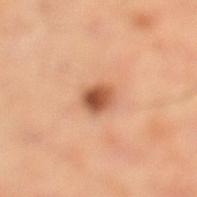biopsy status: total-body-photography surveillance lesion; no biopsy | automated lesion analysis: a footprint of about 5 mm², an eccentricity of roughly 0.6, and two-axis asymmetry of about 0.15; an average lesion color of about L≈56 a*≈26 b*≈36 (CIELAB), roughly 16 lightness units darker than nearby skin, and a normalized lesion–skin contrast near 10; border irregularity of about 1.5 on a 0–10 scale, a within-lesion color-variation index near 6/10, and peripheral color asymmetry of about 2.5; a classifier nevus-likeness of about 95/100 and a lesion-detection confidence of about 100/100 | image: ~15 mm tile from a whole-body skin photo | patient: female, in their 60s | illumination: cross-polarized | lesion diameter: about 2.5 mm | site: the right leg.